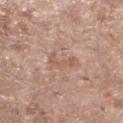Recorded during total-body skin imaging; not selected for excision or biopsy. The lesion is located on the leg. A 15 mm close-up tile from a total-body photography series done for melanoma screening. The subject is a female about 75 years old. Longest diameter approximately 3.5 mm. Captured under white-light illumination. Automated tile analysis of the lesion measured a footprint of about 3.5 mm² and a symmetry-axis asymmetry near 0.65. It also reported a lesion color around L≈57 a*≈19 b*≈28 in CIELAB and a lesion-to-skin contrast of about 5 (normalized; higher = more distinct).